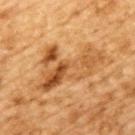biopsy status: catalogued during a skin exam; not biopsied | lighting: cross-polarized | image source: ~15 mm tile from a whole-body skin photo | patient: male, aged 83 or older | body site: the upper back.